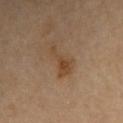Context: About 5 mm across. A male subject about 85 years old or older. The lesion is on the right upper arm. Cropped from a total-body skin-imaging series; the visible field is about 15 mm.